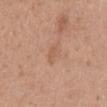<tbp_lesion>
  <image>
    <source>total-body photography crop</source>
    <field_of_view_mm>15</field_of_view_mm>
  </image>
  <lesion_size>
    <long_diameter_mm_approx>3.5</long_diameter_mm_approx>
  </lesion_size>
  <site>mid back</site>
  <lighting>white-light</lighting>
  <patient>
    <sex>female</sex>
    <age_approx>40</age_approx>
  </patient>
</tbp_lesion>